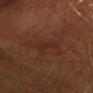notes = total-body-photography surveillance lesion; no biopsy | subject = male, about 60 years old | lighting = cross-polarized illumination | diameter = about 3.5 mm | acquisition = 15 mm crop, total-body photography | site = the head or neck.